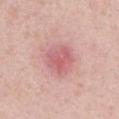<lesion>
  <biopsy_status>not biopsied; imaged during a skin examination</biopsy_status>
  <site>left upper arm</site>
  <lighting>white-light</lighting>
  <patient>
    <sex>male</sex>
    <age_approx>50</age_approx>
  </patient>
  <image>
    <source>total-body photography crop</source>
    <field_of_view_mm>15</field_of_view_mm>
  </image>
</lesion>